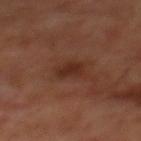follow-up: catalogued during a skin exam; not biopsied | body site: the back | image: 15 mm crop, total-body photography | subject: male, aged 68 to 72 | TBP lesion metrics: a border-irregularity rating of about 2.5/10, a color-variation rating of about 2/10, and peripheral color asymmetry of about 0.5; a classifier nevus-likeness of about 50/100 and a detector confidence of about 100 out of 100 that the crop contains a lesion.